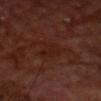Background:
Approximately 3.5 mm at its widest. A male patient, about 70 years old. Captured under cross-polarized illumination. This image is a 15 mm lesion crop taken from a total-body photograph. On the front of the torso.